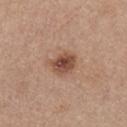Case summary:
* notes: no biopsy performed (imaged during a skin exam)
* body site: the chest
* image: total-body-photography crop, ~15 mm field of view
* size: about 3.5 mm
* subject: female, aged 63–67